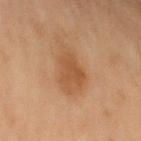Clinical impression: Part of a total-body skin-imaging series; this lesion was reviewed on a skin check and was not flagged for biopsy. Context: Located on the left upper arm. Cropped from a whole-body photographic skin survey; the tile spans about 15 mm. Longest diameter approximately 6 mm. A female subject aged approximately 55. Imaged with cross-polarized lighting.